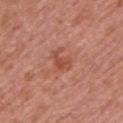<lesion>
<biopsy_status>not biopsied; imaged during a skin examination</biopsy_status>
<patient>
  <sex>male</sex>
  <age_approx>65</age_approx>
</patient>
<site>left upper arm</site>
<image>
  <source>total-body photography crop</source>
  <field_of_view_mm>15</field_of_view_mm>
</image>
</lesion>